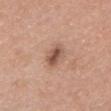{"biopsy_status": "not biopsied; imaged during a skin examination", "site": "head or neck", "patient": {"sex": "female", "age_approx": 65}, "lesion_size": {"long_diameter_mm_approx": 3.5}, "image": {"source": "total-body photography crop", "field_of_view_mm": 15}, "lighting": "white-light", "automated_metrics": {"area_mm2_approx": 5.0, "eccentricity": 0.85, "shape_asymmetry": 0.2, "cielab_L": 53, "cielab_a": 21, "cielab_b": 27, "vs_skin_darker_L": 12.0, "vs_skin_contrast_norm": 8.5, "border_irregularity_0_10": 2.5, "color_variation_0_10": 4.0, "peripheral_color_asymmetry": 1.5}}